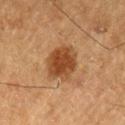workup = no biopsy performed (imaged during a skin exam) | image source = total-body-photography crop, ~15 mm field of view | patient = male, roughly 75 years of age | anatomic site = the left thigh.